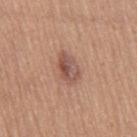| key | value |
|---|---|
| follow-up | no biopsy performed (imaged during a skin exam) |
| anatomic site | the right thigh |
| diameter | ≈4 mm |
| acquisition | 15 mm crop, total-body photography |
| tile lighting | white-light |
| subject | female, aged 53 to 57 |